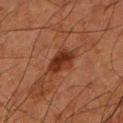Recorded during total-body skin imaging; not selected for excision or biopsy.
Located on the left lower leg.
A roughly 15 mm field-of-view crop from a total-body skin photograph.
The patient is a male aged 78 to 82.